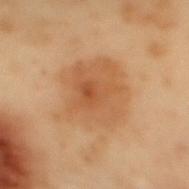Findings:
* biopsy status: imaged on a skin check; not biopsied
* subject: male, in their mid- to late 50s
* TBP lesion metrics: an outline eccentricity of about 0.5 (0 = round, 1 = elongated) and a symmetry-axis asymmetry near 0.2; roughly 7 lightness units darker than nearby skin; internal color variation of about 4 on a 0–10 scale and a peripheral color-asymmetry measure near 1; a classifier nevus-likeness of about 25/100
* location: the mid back
* image: 15 mm crop, total-body photography
* size: ≈6.5 mm